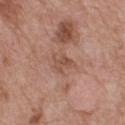Imaged during a routine full-body skin examination; the lesion was not biopsied and no histopathology is available.
Automated image analysis of the tile measured border irregularity of about 4.5 on a 0–10 scale, a within-lesion color-variation index near 2/10, and a peripheral color-asymmetry measure near 1.
About 3 mm across.
This image is a 15 mm lesion crop taken from a total-body photograph.
The tile uses white-light illumination.
The lesion is located on the back.
A male subject in their mid- to late 60s.